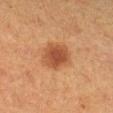{
  "biopsy_status": "not biopsied; imaged during a skin examination",
  "image": {
    "source": "total-body photography crop",
    "field_of_view_mm": 15
  },
  "site": "leg",
  "patient": {
    "sex": "female",
    "age_approx": 40
  }
}